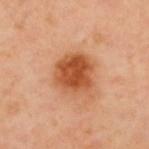Imaged during a routine full-body skin examination; the lesion was not biopsied and no histopathology is available.
The subject is a male aged 63–67.
Located on the upper back.
Captured under cross-polarized illumination.
A roughly 15 mm field-of-view crop from a total-body skin photograph.